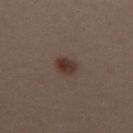Clinical impression: No biopsy was performed on this lesion — it was imaged during a full skin examination and was not determined to be concerning. Context: Automated image analysis of the tile measured an average lesion color of about L≈33 a*≈16 b*≈21 (CIELAB), a lesion–skin lightness drop of about 10, and a lesion-to-skin contrast of about 9.5 (normalized; higher = more distinct). A female patient, approximately 30 years of age. A 15 mm close-up extracted from a 3D total-body photography capture. This is a white-light tile. Located on the upper back. Longest diameter approximately 2.5 mm.